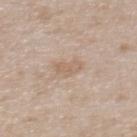Clinical impression: The lesion was tiled from a total-body skin photograph and was not biopsied. Acquisition and patient details: A 15 mm close-up tile from a total-body photography series done for melanoma screening. A female subject aged around 30. The lesion is located on the upper back. Imaged with white-light lighting.